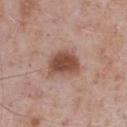imaging modality = 15 mm crop, total-body photography | automated metrics = an area of roughly 9.5 mm² and two-axis asymmetry of about 0.25; a mean CIELAB color near L≈48 a*≈22 b*≈27, about 14 CIELAB-L* units darker than the surrounding skin, and a normalized lesion–skin contrast near 10; an automated nevus-likeness rating near 90 out of 100 and a detector confidence of about 100 out of 100 that the crop contains a lesion | lesion size = about 3.5 mm | location = the chest | subject = male, about 75 years old.